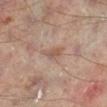- workup · catalogued during a skin exam; not biopsied
- lighting · cross-polarized
- location · the right lower leg
- lesion diameter · ~3 mm (longest diameter)
- patient · male, aged approximately 65
- automated lesion analysis · an eccentricity of roughly 0.8 and a shape-asymmetry score of about 0.4 (0 = symmetric); border irregularity of about 4 on a 0–10 scale, a within-lesion color-variation index near 3.5/10, and radial color variation of about 1; an automated nevus-likeness rating near 0 out of 100 and lesion-presence confidence of about 100/100
- imaging modality · 15 mm crop, total-body photography